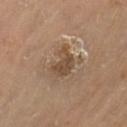| key | value |
|---|---|
| workup | catalogued during a skin exam; not biopsied |
| lesion diameter | ≈4 mm |
| tile lighting | cross-polarized illumination |
| automated lesion analysis | a mean CIELAB color near L≈48 a*≈15 b*≈30, a lesion–skin lightness drop of about 10, and a normalized lesion–skin contrast near 7.5; a nevus-likeness score of about 60/100 and lesion-presence confidence of about 100/100 |
| anatomic site | the left thigh |
| subject | female, aged approximately 70 |
| image | total-body-photography crop, ~15 mm field of view |